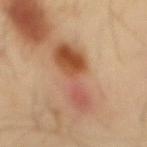size: ~7.5 mm (longest diameter) | tile lighting: cross-polarized | body site: the mid back | image-analysis metrics: lesion-presence confidence of about 100/100 | patient: male, approximately 40 years of age | imaging modality: total-body-photography crop, ~15 mm field of view.